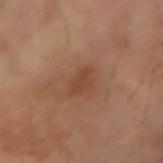Recorded during total-body skin imaging; not selected for excision or biopsy.
The patient is a male aged approximately 70.
The recorded lesion diameter is about 3 mm.
Automated image analysis of the tile measured a footprint of about 3.5 mm², an eccentricity of roughly 0.8, and two-axis asymmetry of about 0.4.
Cropped from a whole-body photographic skin survey; the tile spans about 15 mm.
On the right forearm.